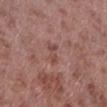Impression:
Part of a total-body skin-imaging series; this lesion was reviewed on a skin check and was not flagged for biopsy.
Image and clinical context:
A roughly 15 mm field-of-view crop from a total-body skin photograph. The lesion-visualizer software estimated border irregularity of about 4 on a 0–10 scale, internal color variation of about 2 on a 0–10 scale, and a peripheral color-asymmetry measure near 0.5. A male patient, about 55 years old. This is a white-light tile. The recorded lesion diameter is about 3 mm. The lesion is on the left lower leg.